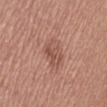The lesion's longest dimension is about 4 mm. Located on the left thigh. Cropped from a total-body skin-imaging series; the visible field is about 15 mm. The subject is a female approximately 25 years of age.